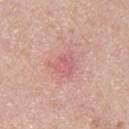| field | value |
|---|---|
| workup | imaged on a skin check; not biopsied |
| tile lighting | white-light illumination |
| location | the upper back |
| diameter | about 3.5 mm |
| patient | male, about 40 years old |
| acquisition | 15 mm crop, total-body photography |